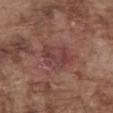* notes — imaged on a skin check; not biopsied
* illumination — white-light
* TBP lesion metrics — a classifier nevus-likeness of about 10/100 and lesion-presence confidence of about 100/100
* patient — male, aged 73–77
* image — 15 mm crop, total-body photography
* body site — the abdomen